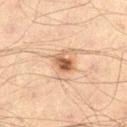| feature | finding |
|---|---|
| workup | total-body-photography surveillance lesion; no biopsy |